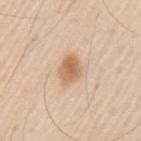{
  "biopsy_status": "not biopsied; imaged during a skin examination",
  "lighting": "white-light",
  "lesion_size": {
    "long_diameter_mm_approx": 3.5
  },
  "site": "arm",
  "patient": {
    "sex": "male",
    "age_approx": 60
  },
  "image": {
    "source": "total-body photography crop",
    "field_of_view_mm": 15
  }
}